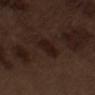Q: Was a biopsy performed?
A: imaged on a skin check; not biopsied
Q: Automated lesion metrics?
A: a lesion area of about 6 mm², an outline eccentricity of about 0.7 (0 = round, 1 = elongated), and a shape-asymmetry score of about 0.25 (0 = symmetric); a lesion–skin lightness drop of about 6 and a normalized border contrast of about 9; border irregularity of about 2 on a 0–10 scale, a color-variation rating of about 1.5/10, and a peripheral color-asymmetry measure near 0.5; an automated nevus-likeness rating near 50 out of 100 and a lesion-detection confidence of about 100/100
Q: Patient demographics?
A: male, aged 68–72
Q: How large is the lesion?
A: ~3 mm (longest diameter)
Q: What kind of image is this?
A: ~15 mm crop, total-body skin-cancer survey
Q: Lesion location?
A: the left upper arm
Q: What lighting was used for the tile?
A: white-light illumination